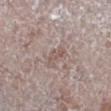biopsy status: total-body-photography surveillance lesion; no biopsy
image source: ~15 mm tile from a whole-body skin photo
TBP lesion metrics: a lesion-to-skin contrast of about 5.5 (normalized; higher = more distinct); a nevus-likeness score of about 0/100 and a lesion-detection confidence of about 60/100
lesion diameter: about 2.5 mm
site: the right leg
patient: male, approximately 70 years of age